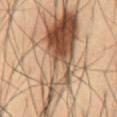{"biopsy_status": "not biopsied; imaged during a skin examination", "image": {"source": "total-body photography crop", "field_of_view_mm": 15}, "site": "abdomen", "lesion_size": {"long_diameter_mm_approx": 14.5}, "patient": {"sex": "male", "age_approx": 55}, "lighting": "cross-polarized"}